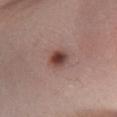notes: catalogued during a skin exam; not biopsied
subject: female, about 35 years old
image: ~15 mm tile from a whole-body skin photo
site: the leg
automated lesion analysis: a footprint of about 5 mm² and a symmetry-axis asymmetry near 0.15
lesion size: ~2.5 mm (longest diameter)
tile lighting: white-light